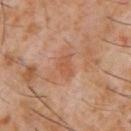Part of a total-body skin-imaging series; this lesion was reviewed on a skin check and was not flagged for biopsy.
The lesion is located on the chest.
The subject is a male aged 58 to 62.
A 15 mm crop from a total-body photograph taken for skin-cancer surveillance.
Captured under cross-polarized illumination.